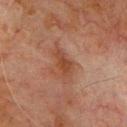Imaged during a routine full-body skin examination; the lesion was not biopsied and no histopathology is available.
Measured at roughly 4 mm in maximum diameter.
Cropped from a whole-body photographic skin survey; the tile spans about 15 mm.
The lesion is located on the chest.
The subject is a male approximately 80 years of age.